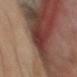Impression:
Recorded during total-body skin imaging; not selected for excision or biopsy.
Image and clinical context:
A male patient aged around 50. The tile uses cross-polarized illumination. The lesion's longest dimension is about 4 mm. An algorithmic analysis of the crop reported roughly 7 lightness units darker than nearby skin. And it measured a nevus-likeness score of about 0/100 and a lesion-detection confidence of about 65/100. Cropped from a total-body skin-imaging series; the visible field is about 15 mm. From the head or neck.